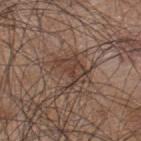The lesion was photographed on a routine skin check and not biopsied; there is no pathology result. The tile uses white-light illumination. A male patient, in their mid-40s. The recorded lesion diameter is about 4 mm. Automated tile analysis of the lesion measured about 8 CIELAB-L* units darker than the surrounding skin and a normalized border contrast of about 7. And it measured a border-irregularity rating of about 9/10, a color-variation rating of about 2.5/10, and radial color variation of about 0.5. A 15 mm close-up extracted from a 3D total-body photography capture. On the upper back.